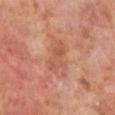{
  "biopsy_status": "not biopsied; imaged during a skin examination",
  "lesion_size": {
    "long_diameter_mm_approx": 4.5
  },
  "automated_metrics": {
    "area_mm2_approx": 7.0,
    "eccentricity": 0.9,
    "cielab_L": 53,
    "cielab_a": 24,
    "cielab_b": 32,
    "vs_skin_darker_L": 7.0,
    "vs_skin_contrast_norm": 5.5,
    "border_irregularity_0_10": 4.5,
    "color_variation_0_10": 4.0,
    "lesion_detection_confidence_0_100": 100
  },
  "patient": {
    "sex": "male",
    "age_approx": 70
  },
  "site": "left lower leg",
  "image": {
    "source": "total-body photography crop",
    "field_of_view_mm": 15
  }
}